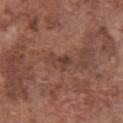workup: total-body-photography surveillance lesion; no biopsy
patient: male, approximately 75 years of age
image-analysis metrics: a lesion color around L≈40 a*≈20 b*≈24 in CIELAB and a lesion-to-skin contrast of about 6.5 (normalized; higher = more distinct); border irregularity of about 5.5 on a 0–10 scale and a color-variation rating of about 2/10; an automated nevus-likeness rating near 5 out of 100
lesion diameter: about 3 mm
anatomic site: the chest
image: 15 mm crop, total-body photography
lighting: white-light illumination